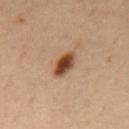Q: What is the anatomic site?
A: the mid back
Q: What lighting was used for the tile?
A: cross-polarized illumination
Q: What is the imaging modality?
A: total-body-photography crop, ~15 mm field of view
Q: How large is the lesion?
A: ≈3.5 mm
Q: What are the patient's age and sex?
A: male, in their mid- to late 50s